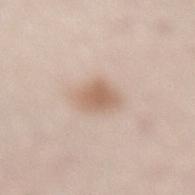The lesion was tiled from a total-body skin photograph and was not biopsied. A female subject, roughly 50 years of age. A region of skin cropped from a whole-body photographic capture, roughly 15 mm wide. On the lower back. Captured under white-light illumination. Measured at roughly 4 mm in maximum diameter. The lesion-visualizer software estimated a nevus-likeness score of about 90/100 and a detector confidence of about 100 out of 100 that the crop contains a lesion.